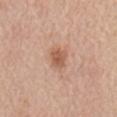workup=imaged on a skin check; not biopsied
image=~15 mm crop, total-body skin-cancer survey
location=the mid back
subject=male, in their mid-60s